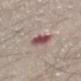Q: What kind of image is this?
A: ~15 mm crop, total-body skin-cancer survey
Q: What is the anatomic site?
A: the mid back
Q: What did automated image analysis measure?
A: a lesion area of about 6 mm² and an outline eccentricity of about 0.2 (0 = round, 1 = elongated); a border-irregularity rating of about 2/10 and radial color variation of about 1.5; an automated nevus-likeness rating near 0 out of 100 and a lesion-detection confidence of about 100/100
Q: What lighting was used for the tile?
A: white-light illumination
Q: Patient demographics?
A: male, about 45 years old